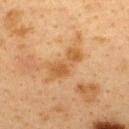A region of skin cropped from a whole-body photographic capture, roughly 15 mm wide. This is a cross-polarized tile. The lesion is located on the back. The subject is a female about 40 years old. Longest diameter approximately 5.5 mm.